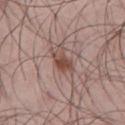Imaged during a routine full-body skin examination; the lesion was not biopsied and no histopathology is available.
Automated tile analysis of the lesion measured a lesion color around L≈46 a*≈20 b*≈24 in CIELAB, roughly 11 lightness units darker than nearby skin, and a normalized lesion–skin contrast near 9. And it measured a border-irregularity index near 3/10.
From the mid back.
A male subject roughly 45 years of age.
Captured under white-light illumination.
A 15 mm close-up tile from a total-body photography series done for melanoma screening.